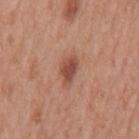Findings:
* workup · total-body-photography surveillance lesion; no biopsy
* patient · male, in their 70s
* site · the mid back
* image · 15 mm crop, total-body photography